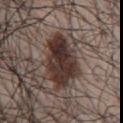Clinical impression:
Captured during whole-body skin photography for melanoma surveillance; the lesion was not biopsied.
Background:
From the chest. The recorded lesion diameter is about 7 mm. A 15 mm close-up extracted from a 3D total-body photography capture. A male patient roughly 70 years of age.